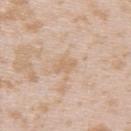Image and clinical context:
A female patient in their mid- to late 20s. A close-up tile cropped from a whole-body skin photograph, about 15 mm across. From the upper back.